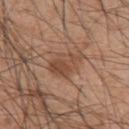Assessment: Recorded during total-body skin imaging; not selected for excision or biopsy. Image and clinical context: The patient is a male aged around 45. Imaged with white-light lighting. Located on the upper back. Longest diameter approximately 4.5 mm. A lesion tile, about 15 mm wide, cut from a 3D total-body photograph.